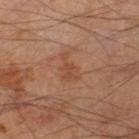notes: total-body-photography surveillance lesion; no biopsy | patient: male, aged approximately 65 | location: the right lower leg | imaging modality: 15 mm crop, total-body photography.